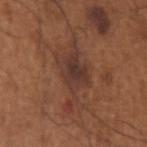anatomic site=the left upper arm
image source=total-body-photography crop, ~15 mm field of view
patient=male, aged around 65
automated metrics=a mean CIELAB color near L≈36 a*≈20 b*≈25; a border-irregularity index near 7.5/10 and a within-lesion color-variation index near 4/10
lesion diameter=~6.5 mm (longest diameter)
tile lighting=white-light